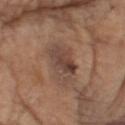illumination — white-light illumination
subject — male, aged 78–82
automated lesion analysis — a footprint of about 10 mm², an eccentricity of roughly 0.65, and a shape-asymmetry score of about 0.25 (0 = symmetric); a border-irregularity rating of about 3.5/10, a color-variation rating of about 6.5/10, and peripheral color asymmetry of about 2.5; a classifier nevus-likeness of about 10/100 and a lesion-detection confidence of about 100/100
site — the head or neck
acquisition — 15 mm crop, total-body photography